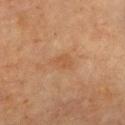Assessment:
The lesion was tiled from a total-body skin photograph and was not biopsied.
Context:
Approximately 2.5 mm at its widest. The lesion-visualizer software estimated a lesion color around L≈47 a*≈20 b*≈33 in CIELAB, a lesion–skin lightness drop of about 5, and a normalized border contrast of about 5. The analysis additionally found a peripheral color-asymmetry measure near 0.5. The software also gave a nevus-likeness score of about 0/100. This is a cross-polarized tile. A female subject, aged approximately 60. A lesion tile, about 15 mm wide, cut from a 3D total-body photograph. On the chest.